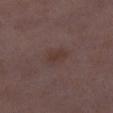No biopsy was performed on this lesion — it was imaged during a full skin examination and was not determined to be concerning.
A female subject in their 30s.
The lesion-visualizer software estimated a lesion area of about 3.5 mm², an eccentricity of roughly 0.75, and a shape-asymmetry score of about 0.25 (0 = symmetric). The software also gave a lesion–skin lightness drop of about 6. It also reported internal color variation of about 1.5 on a 0–10 scale.
The lesion is on the right lower leg.
Approximately 2.5 mm at its widest.
A 15 mm close-up extracted from a 3D total-body photography capture.
This is a white-light tile.